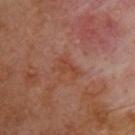{
  "biopsy_status": "not biopsied; imaged during a skin examination",
  "lighting": "cross-polarized",
  "lesion_size": {
    "long_diameter_mm_approx": 2.5
  },
  "patient": {
    "sex": "male",
    "age_approx": 70
  },
  "site": "upper back",
  "image": {
    "source": "total-body photography crop",
    "field_of_view_mm": 15
  }
}